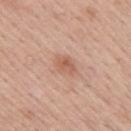Impression:
Part of a total-body skin-imaging series; this lesion was reviewed on a skin check and was not flagged for biopsy.